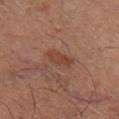Background:
The lesion is on the right lower leg. The subject is roughly 65 years of age. A close-up tile cropped from a whole-body skin photograph, about 15 mm across.